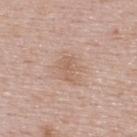<case>
  <biopsy_status>not biopsied; imaged during a skin examination</biopsy_status>
  <patient>
    <sex>female</sex>
    <age_approx>50</age_approx>
  </patient>
  <image>
    <source>total-body photography crop</source>
    <field_of_view_mm>15</field_of_view_mm>
  </image>
  <site>upper back</site>
</case>